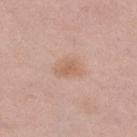Part of a total-body skin-imaging series; this lesion was reviewed on a skin check and was not flagged for biopsy.
Approximately 3 mm at its widest.
This is a white-light tile.
A region of skin cropped from a whole-body photographic capture, roughly 15 mm wide.
The lesion is located on the right lower leg.
A female subject, approximately 65 years of age.
Automated image analysis of the tile measured a nevus-likeness score of about 10/100.